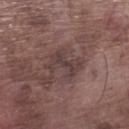Q: Who is the patient?
A: male, aged 73–77
Q: Lesion location?
A: the left lower leg
Q: What kind of image is this?
A: ~15 mm crop, total-body skin-cancer survey